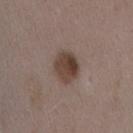- follow-up — no biopsy performed (imaged during a skin exam)